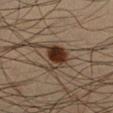The lesion was tiled from a total-body skin photograph and was not biopsied. Captured under cross-polarized illumination. From the left lower leg. A male patient aged approximately 35. Approximately 3.5 mm at its widest. Cropped from a whole-body photographic skin survey; the tile spans about 15 mm. Automated tile analysis of the lesion measured an area of roughly 7.5 mm², a shape eccentricity near 0.6, and a symmetry-axis asymmetry near 0.3. It also reported a mean CIELAB color near L≈28 a*≈15 b*≈23, roughly 14 lightness units darker than nearby skin, and a normalized border contrast of about 13.5. And it measured a border-irregularity index near 2.5/10, internal color variation of about 7.5 on a 0–10 scale, and a peripheral color-asymmetry measure near 2.5.